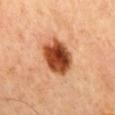notes — imaged on a skin check; not biopsied
image-analysis metrics — an area of roughly 15 mm², an eccentricity of roughly 0.65, and a shape-asymmetry score of about 0.1 (0 = symmetric); a mean CIELAB color near L≈49 a*≈29 b*≈38, about 21 CIELAB-L* units darker than the surrounding skin, and a lesion-to-skin contrast of about 13.5 (normalized; higher = more distinct); a color-variation rating of about 9/10 and peripheral color asymmetry of about 3; a classifier nevus-likeness of about 100/100 and lesion-presence confidence of about 100/100
illumination — cross-polarized
imaging modality — total-body-photography crop, ~15 mm field of view
subject — male, aged approximately 55
lesion size — ≈5 mm
body site — the mid back